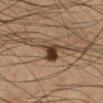| feature | finding |
|---|---|
| biopsy status | catalogued during a skin exam; not biopsied |
| subject | male, in their mid-30s |
| image source | 15 mm crop, total-body photography |
| location | the leg |